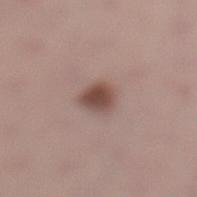Imaged during a routine full-body skin examination; the lesion was not biopsied and no histopathology is available. Measured at roughly 3 mm in maximum diameter. The lesion is on the left lower leg. This image is a 15 mm lesion crop taken from a total-body photograph. This is a white-light tile. The subject is a female aged 23–27.